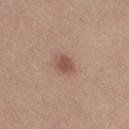Notes:
• lesion size — ~3 mm (longest diameter)
• image source — ~15 mm tile from a whole-body skin photo
• TBP lesion metrics — a lesion color around L≈51 a*≈20 b*≈27 in CIELAB and roughly 10 lightness units darker than nearby skin; border irregularity of about 1.5 on a 0–10 scale, internal color variation of about 2 on a 0–10 scale, and radial color variation of about 0.5; a lesion-detection confidence of about 100/100
• tile lighting — white-light illumination
• subject — female, about 30 years old
• body site — the leg